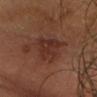Imaged during a routine full-body skin examination; the lesion was not biopsied and no histopathology is available. The subject is a male in their mid- to late 60s. Captured under cross-polarized illumination. A lesion tile, about 15 mm wide, cut from a 3D total-body photograph. From the head or neck.